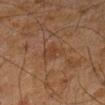notes: imaged on a skin check; not biopsied
size: ≈2.5 mm
site: the right forearm
patient: male, about 45 years old
illumination: cross-polarized illumination
image: 15 mm crop, total-body photography